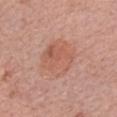{"biopsy_status": "not biopsied; imaged during a skin examination", "patient": {"sex": "female", "age_approx": 65}, "site": "right forearm", "image": {"source": "total-body photography crop", "field_of_view_mm": 15}}